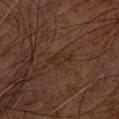biopsy_status: not biopsied; imaged during a skin examination
lesion_size:
  long_diameter_mm_approx: 3.5
image:
  source: total-body photography crop
  field_of_view_mm: 15
site: arm
lighting: cross-polarized
patient:
  sex: male
  age_approx: 80